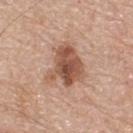Recorded during total-body skin imaging; not selected for excision or biopsy. A male patient in their 70s. A 15 mm close-up tile from a total-body photography series done for melanoma screening. This is a white-light tile. On the upper back.